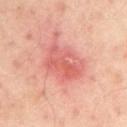Clinical impression: Recorded during total-body skin imaging; not selected for excision or biopsy. Context: Approximately 5.5 mm at its widest. Automated tile analysis of the lesion measured an outline eccentricity of about 0.75 (0 = round, 1 = elongated) and a shape-asymmetry score of about 0.25 (0 = symmetric). The analysis additionally found a border-irregularity rating of about 2.5/10, a color-variation rating of about 5.5/10, and radial color variation of about 2. And it measured a nevus-likeness score of about 20/100 and a detector confidence of about 100 out of 100 that the crop contains a lesion. The lesion is located on the chest. A male subject, aged around 50. This is a cross-polarized tile. A region of skin cropped from a whole-body photographic capture, roughly 15 mm wide.